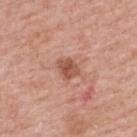Recorded during total-body skin imaging; not selected for excision or biopsy.
Imaged with white-light lighting.
The recorded lesion diameter is about 2.5 mm.
The patient is a female in their 60s.
From the upper back.
A 15 mm crop from a total-body photograph taken for skin-cancer surveillance.